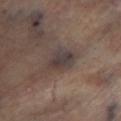Case summary:
• follow-up · catalogued during a skin exam; not biopsied
• lesion size · ≈3.5 mm
• image source · 15 mm crop, total-body photography
• tile lighting · cross-polarized illumination
• site · the left lower leg
• automated lesion analysis · an area of roughly 7 mm², an outline eccentricity of about 0.75 (0 = round, 1 = elongated), and two-axis asymmetry of about 0.25; a border-irregularity rating of about 2.5/10, internal color variation of about 3.5 on a 0–10 scale, and peripheral color asymmetry of about 1; a classifier nevus-likeness of about 0/100